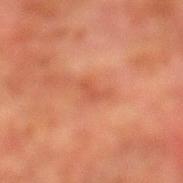imaging modality: ~15 mm tile from a whole-body skin photo | patient: male, aged 73–77 | illumination: cross-polarized | size: ≈2.5 mm | site: the left lower leg | TBP lesion metrics: a mean CIELAB color near L≈45 a*≈27 b*≈31, roughly 6 lightness units darker than nearby skin, and a normalized border contrast of about 5.